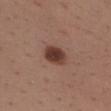notes: no biopsy performed (imaged during a skin exam)
lesion size: ~3 mm (longest diameter)
anatomic site: the upper back
patient: male, about 40 years old
image: 15 mm crop, total-body photography
TBP lesion metrics: an area of roughly 6.5 mm², an outline eccentricity of about 0.65 (0 = round, 1 = elongated), and two-axis asymmetry of about 0.2; a mean CIELAB color near L≈38 a*≈20 b*≈25, a lesion–skin lightness drop of about 14, and a lesion-to-skin contrast of about 11 (normalized; higher = more distinct); a border-irregularity index near 1.5/10 and a within-lesion color-variation index near 4/10; lesion-presence confidence of about 100/100
lighting: white-light